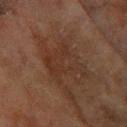biopsy status — imaged on a skin check; not biopsied | lesion size — ~5.5 mm (longest diameter) | illumination — cross-polarized | patient — female, aged approximately 80 | location — the right forearm | image source — ~15 mm crop, total-body skin-cancer survey.